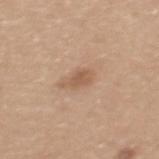No biopsy was performed on this lesion — it was imaged during a full skin examination and was not determined to be concerning. The lesion's longest dimension is about 3 mm. The lesion is on the upper back. A male patient, approximately 40 years of age. A close-up tile cropped from a whole-body skin photograph, about 15 mm across. The tile uses white-light illumination.